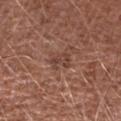| field | value |
|---|---|
| follow-up | no biopsy performed (imaged during a skin exam) |
| automated metrics | a footprint of about 4.5 mm², an outline eccentricity of about 0.8 (0 = round, 1 = elongated), and two-axis asymmetry of about 0.3; a border-irregularity index near 3.5/10, a color-variation rating of about 2.5/10, and peripheral color asymmetry of about 1 |
| anatomic site | the right upper arm |
| image source | total-body-photography crop, ~15 mm field of view |
| subject | male, about 45 years old |
| tile lighting | white-light |